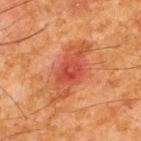The lesion was photographed on a routine skin check and not biopsied; there is no pathology result. Captured under cross-polarized illumination. An algorithmic analysis of the crop reported a lesion area of about 16 mm² and a shape-asymmetry score of about 0.3 (0 = symmetric). And it measured an automated nevus-likeness rating near 90 out of 100 and a lesion-detection confidence of about 100/100. On the back. A region of skin cropped from a whole-body photographic capture, roughly 15 mm wide. A male patient, aged approximately 65.